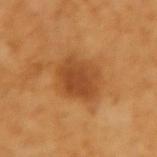Part of a total-body skin-imaging series; this lesion was reviewed on a skin check and was not flagged for biopsy. Measured at roughly 4.5 mm in maximum diameter. A female patient, in their mid-50s. On the left forearm. A roughly 15 mm field-of-view crop from a total-body skin photograph.